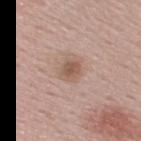Clinical impression:
Captured during whole-body skin photography for melanoma surveillance; the lesion was not biopsied.
Clinical summary:
Automated image analysis of the tile measured an eccentricity of roughly 0.6. The analysis additionally found an average lesion color of about L≈55 a*≈18 b*≈27 (CIELAB), roughly 9 lightness units darker than nearby skin, and a normalized lesion–skin contrast near 7. It also reported a border-irregularity index near 1.5/10, a within-lesion color-variation index near 3/10, and radial color variation of about 1. Cropped from a total-body skin-imaging series; the visible field is about 15 mm. A male patient about 60 years old. The recorded lesion diameter is about 2.5 mm. This is a white-light tile. On the right upper arm.